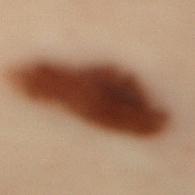<lesion>
<patient>
  <sex>female</sex>
  <age_approx>60</age_approx>
</patient>
<automated_metrics>
  <area_mm2_approx>55.0</area_mm2_approx>
  <eccentricity>0.9</eccentricity>
</automated_metrics>
<lesion_size>
  <long_diameter_mm_approx>13.0</long_diameter_mm_approx>
</lesion_size>
<image>
  <source>total-body photography crop</source>
  <field_of_view_mm>15</field_of_view_mm>
</image>
<lighting>cross-polarized</lighting>
<site>mid back</site>
</lesion>